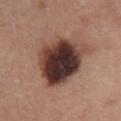Image and clinical context: Automated image analysis of the tile measured a lesion area of about 27 mm². The analysis additionally found a mean CIELAB color near L≈36 a*≈19 b*≈22, roughly 21 lightness units darker than nearby skin, and a lesion-to-skin contrast of about 16 (normalized; higher = more distinct). The analysis additionally found a border-irregularity rating of about 2/10, a color-variation rating of about 10/10, and peripheral color asymmetry of about 3.5. On the chest. The patient is a female in their 50s. A region of skin cropped from a whole-body photographic capture, roughly 15 mm wide. This is a white-light tile. Approximately 7 mm at its widest.